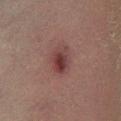Q: Was a biopsy performed?
A: imaged on a skin check; not biopsied
Q: Where on the body is the lesion?
A: the left lower leg
Q: What is the imaging modality?
A: 15 mm crop, total-body photography
Q: Patient demographics?
A: male, roughly 70 years of age